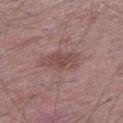  biopsy_status: not biopsied; imaged during a skin examination
  patient:
    sex: male
    age_approx: 50
  lesion_size:
    long_diameter_mm_approx: 4.5
  image:
    source: total-body photography crop
    field_of_view_mm: 15
  lighting: white-light
  site: left lower leg
  automated_metrics:
    area_mm2_approx: 9.0
    eccentricity: 0.85
    shape_asymmetry: 0.3
    nevus_likeness_0_100: 0
    lesion_detection_confidence_0_100: 100